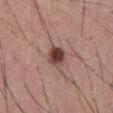follow-up: total-body-photography surveillance lesion; no biopsy
patient: male, aged around 40
location: the abdomen
imaging modality: ~15 mm crop, total-body skin-cancer survey
diameter: ~3 mm (longest diameter)